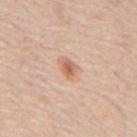biopsy status — catalogued during a skin exam; not biopsied
image-analysis metrics — an automated nevus-likeness rating near 85 out of 100
image — 15 mm crop, total-body photography
illumination — white-light illumination
site — the mid back
lesion diameter — ~2.5 mm (longest diameter)
patient — male, aged 73–77